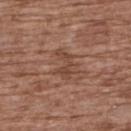Captured during whole-body skin photography for melanoma surveillance; the lesion was not biopsied.
Located on the upper back.
A female subject approximately 75 years of age.
An algorithmic analysis of the crop reported a footprint of about 6 mm², a shape eccentricity near 0.75, and two-axis asymmetry of about 0.65. And it measured internal color variation of about 2.5 on a 0–10 scale and a peripheral color-asymmetry measure near 1. It also reported a nevus-likeness score of about 0/100 and lesion-presence confidence of about 100/100.
A 15 mm close-up tile from a total-body photography series done for melanoma screening.
Approximately 3.5 mm at its widest.
This is a white-light tile.